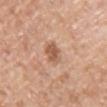Q: Where on the body is the lesion?
A: the chest
Q: How was the tile lit?
A: white-light illumination
Q: How was this image acquired?
A: ~15 mm crop, total-body skin-cancer survey
Q: Lesion size?
A: ≈3 mm
Q: Patient demographics?
A: male, approximately 40 years of age